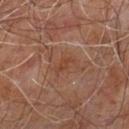Q: Was a biopsy performed?
A: no biopsy performed (imaged during a skin exam)
Q: How was the tile lit?
A: cross-polarized
Q: Lesion location?
A: the right leg
Q: How was this image acquired?
A: ~15 mm tile from a whole-body skin photo
Q: Lesion size?
A: ~2.5 mm (longest diameter)
Q: Patient demographics?
A: male, roughly 60 years of age